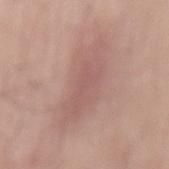notes: catalogued during a skin exam; not biopsied | location: the mid back | subject: male, about 70 years old | image source: total-body-photography crop, ~15 mm field of view | lesion size: ≈9 mm | illumination: white-light.